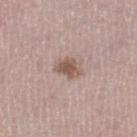The lesion was photographed on a routine skin check and not biopsied; there is no pathology result.
The tile uses white-light illumination.
About 3.5 mm across.
On the leg.
The lesion-visualizer software estimated a lesion color around L≈54 a*≈17 b*≈23 in CIELAB, a lesion–skin lightness drop of about 12, and a normalized lesion–skin contrast near 8. The analysis additionally found a border-irregularity rating of about 3/10, a color-variation rating of about 2.5/10, and radial color variation of about 1. The analysis additionally found lesion-presence confidence of about 100/100.
A female subject aged around 50.
A 15 mm close-up tile from a total-body photography series done for melanoma screening.